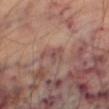Assessment: The lesion was tiled from a total-body skin photograph and was not biopsied. Background: The subject is a male aged 68 to 72. A 15 mm close-up tile from a total-body photography series done for melanoma screening. Automated image analysis of the tile measured an eccentricity of roughly 0.7. And it measured an automated nevus-likeness rating near 0 out of 100 and a detector confidence of about 80 out of 100 that the crop contains a lesion. Located on the right thigh. This is a cross-polarized tile. The recorded lesion diameter is about 3 mm.